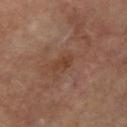{"lighting": "cross-polarized", "lesion_size": {"long_diameter_mm_approx": 2.5}, "automated_metrics": {"cielab_L": 41, "cielab_a": 21, "cielab_b": 30, "vs_skin_darker_L": 6.0, "vs_skin_contrast_norm": 6.5, "border_irregularity_0_10": 3.5, "lesion_detection_confidence_0_100": 100}, "site": "right forearm", "patient": {"sex": "female", "age_approx": 65}, "image": {"source": "total-body photography crop", "field_of_view_mm": 15}}